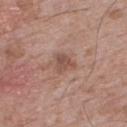imaging modality: ~15 mm crop, total-body skin-cancer survey | tile lighting: white-light | subject: male, in their 70s | body site: the upper back | lesion size: ≈3 mm.